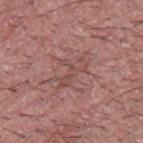No biopsy was performed on this lesion — it was imaged during a full skin examination and was not determined to be concerning. This image is a 15 mm lesion crop taken from a total-body photograph. Longest diameter approximately 4.5 mm. Imaged with white-light lighting. The lesion-visualizer software estimated a lesion color around L≈49 a*≈22 b*≈23 in CIELAB, about 6 CIELAB-L* units darker than the surrounding skin, and a normalized border contrast of about 5. The software also gave a border-irregularity rating of about 8/10, a within-lesion color-variation index near 2.5/10, and peripheral color asymmetry of about 1. The software also gave a nevus-likeness score of about 0/100 and a detector confidence of about 55 out of 100 that the crop contains a lesion. From the chest. A male subject, roughly 40 years of age.